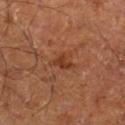{"biopsy_status": "not biopsied; imaged during a skin examination", "patient": {"age_approx": 65}, "image": {"source": "total-body photography crop", "field_of_view_mm": 15}, "lesion_size": {"long_diameter_mm_approx": 3.0}, "site": "right lower leg", "lighting": "cross-polarized"}